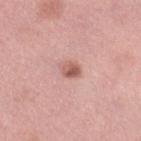Q: What are the patient's age and sex?
A: female, in their 50s
Q: What kind of image is this?
A: ~15 mm crop, total-body skin-cancer survey
Q: Where on the body is the lesion?
A: the right lower leg
Q: Illumination type?
A: white-light illumination